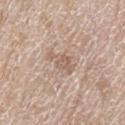The lesion was photographed on a routine skin check and not biopsied; there is no pathology result. A 15 mm close-up tile from a total-body photography series done for melanoma screening. Located on the left thigh. The subject is a male approximately 80 years of age.